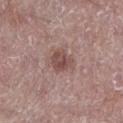Findings:
- notes — total-body-photography surveillance lesion; no biopsy
- patient — female, aged around 65
- tile lighting — white-light
- body site — the left lower leg
- image — total-body-photography crop, ~15 mm field of view
- diameter — ~3 mm (longest diameter)